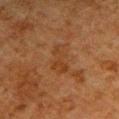Findings:
– automated metrics: an area of roughly 6.5 mm², an eccentricity of roughly 0.9, and two-axis asymmetry of about 0.35; internal color variation of about 2.5 on a 0–10 scale; a detector confidence of about 100 out of 100 that the crop contains a lesion
– imaging modality: total-body-photography crop, ~15 mm field of view
– patient: male, in their 80s
– location: the right upper arm
– lighting: cross-polarized
– diameter: ~4.5 mm (longest diameter)